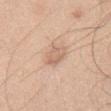Assessment: Imaged during a routine full-body skin examination; the lesion was not biopsied and no histopathology is available. Background: The patient is a male aged around 45. Located on the chest. About 3 mm across. Automated tile analysis of the lesion measured an average lesion color of about L≈65 a*≈19 b*≈30 (CIELAB), a lesion–skin lightness drop of about 8, and a normalized border contrast of about 5.5. The analysis additionally found border irregularity of about 1.5 on a 0–10 scale, internal color variation of about 3 on a 0–10 scale, and radial color variation of about 1. A region of skin cropped from a whole-body photographic capture, roughly 15 mm wide.